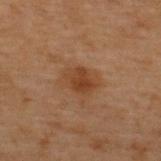Assessment: Recorded during total-body skin imaging; not selected for excision or biopsy. Image and clinical context: A 15 mm crop from a total-body photograph taken for skin-cancer surveillance. Captured under cross-polarized illumination. Longest diameter approximately 3.5 mm. A male subject about 70 years old. An algorithmic analysis of the crop reported a classifier nevus-likeness of about 70/100 and lesion-presence confidence of about 100/100. From the back.